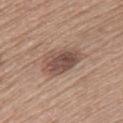This lesion was catalogued during total-body skin photography and was not selected for biopsy. The lesion is located on the upper back. Automated tile analysis of the lesion measured a footprint of about 12 mm² and two-axis asymmetry of about 0.25. The analysis additionally found border irregularity of about 3 on a 0–10 scale, a within-lesion color-variation index near 5/10, and a peripheral color-asymmetry measure near 2. A female patient, about 60 years old. The recorded lesion diameter is about 5 mm. This is a white-light tile. A 15 mm close-up tile from a total-body photography series done for melanoma screening.